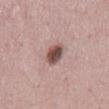Assessment: No biopsy was performed on this lesion — it was imaged during a full skin examination and was not determined to be concerning. Acquisition and patient details: The lesion-visualizer software estimated an area of roughly 5.5 mm², an eccentricity of roughly 0.8, and two-axis asymmetry of about 0.2. It also reported border irregularity of about 2 on a 0–10 scale. The software also gave a nevus-likeness score of about 95/100. A male patient, aged around 50. Measured at roughly 3.5 mm in maximum diameter. On the mid back. Captured under white-light illumination. A 15 mm close-up tile from a total-body photography series done for melanoma screening.